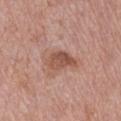This lesion was catalogued during total-body skin photography and was not selected for biopsy. A male subject, in their 70s. From the right upper arm. Captured under white-light illumination. Cropped from a whole-body photographic skin survey; the tile spans about 15 mm.